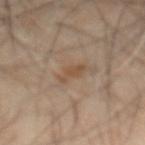Captured under cross-polarized illumination. Located on the lower back. A male patient aged around 60. A roughly 15 mm field-of-view crop from a total-body skin photograph. The lesion's longest dimension is about 3 mm.